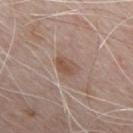Q: What is the lesion's diameter?
A: ≈3 mm
Q: How was the tile lit?
A: white-light illumination
Q: What are the patient's age and sex?
A: male, aged 68–72
Q: What is the imaging modality?
A: ~15 mm crop, total-body skin-cancer survey
Q: Lesion location?
A: the front of the torso